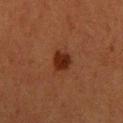The lesion was photographed on a routine skin check and not biopsied; there is no pathology result. A female subject, aged 38 to 42. A 15 mm close-up extracted from a 3D total-body photography capture. Located on the upper back.